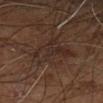The patient is a male approximately 60 years of age.
Automated image analysis of the tile measured a lesion color around L≈26 a*≈14 b*≈20 in CIELAB, roughly 5 lightness units darker than nearby skin, and a normalized border contrast of about 6. The analysis additionally found a border-irregularity rating of about 6/10, a color-variation rating of about 3.5/10, and radial color variation of about 1.5. And it measured a classifier nevus-likeness of about 0/100.
Located on the right leg.
A 15 mm close-up extracted from a 3D total-body photography capture.
Captured under cross-polarized illumination.
Longest diameter approximately 6 mm.